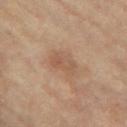The lesion was photographed on a routine skin check and not biopsied; there is no pathology result.
A female subject, roughly 75 years of age.
The lesion-visualizer software estimated an area of roughly 7.5 mm², a shape eccentricity near 0.75, and two-axis asymmetry of about 0.2.
A region of skin cropped from a whole-body photographic capture, roughly 15 mm wide.
The lesion is on the right thigh.